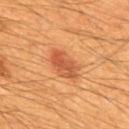Case summary:
– biopsy status — imaged on a skin check; not biopsied
– size — ≈4.5 mm
– imaging modality — ~15 mm crop, total-body skin-cancer survey
– anatomic site — the mid back
– patient — male, roughly 60 years of age
– lighting — cross-polarized illumination
– TBP lesion metrics — border irregularity of about 2 on a 0–10 scale, a within-lesion color-variation index near 5.5/10, and peripheral color asymmetry of about 2; a lesion-detection confidence of about 100/100